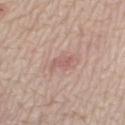Part of a total-body skin-imaging series; this lesion was reviewed on a skin check and was not flagged for biopsy. On the right lower leg. A close-up tile cropped from a whole-body skin photograph, about 15 mm across. A female subject, in their 60s.